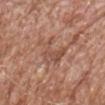{
  "biopsy_status": "not biopsied; imaged during a skin examination",
  "site": "head or neck",
  "patient": {
    "sex": "male",
    "age_approx": 80
  },
  "automated_metrics": {
    "area_mm2_approx": 9.0,
    "eccentricity": 0.65,
    "shape_asymmetry": 0.5,
    "cielab_L": 51,
    "cielab_a": 21,
    "cielab_b": 29,
    "vs_skin_darker_L": 8.0,
    "vs_skin_contrast_norm": 5.5,
    "border_irregularity_0_10": 7.5,
    "color_variation_0_10": 3.5,
    "peripheral_color_asymmetry": 1.0
  },
  "image": {
    "source": "total-body photography crop",
    "field_of_view_mm": 15
  },
  "lighting": "white-light"
}